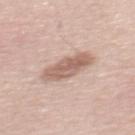follow-up=no biopsy performed (imaged during a skin exam) | location=the back | imaging modality=total-body-photography crop, ~15 mm field of view | lesion diameter=≈5.5 mm | subject=male, aged 53–57 | image-analysis metrics=a footprint of about 11 mm² and two-axis asymmetry of about 0.15; a lesion color around L≈61 a*≈18 b*≈26 in CIELAB, roughly 12 lightness units darker than nearby skin, and a normalized lesion–skin contrast near 8; border irregularity of about 2 on a 0–10 scale, a color-variation rating of about 3.5/10, and radial color variation of about 1; an automated nevus-likeness rating near 10 out of 100 and a detector confidence of about 100 out of 100 that the crop contains a lesion | lighting=white-light illumination.